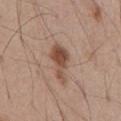This lesion was catalogued during total-body skin photography and was not selected for biopsy. A 15 mm close-up extracted from a 3D total-body photography capture. This is a white-light tile. The patient is a male aged 58 to 62. On the abdomen. Automated image analysis of the tile measured a mean CIELAB color near L≈49 a*≈20 b*≈28 and a normalized lesion–skin contrast near 9. And it measured a border-irregularity rating of about 5/10, a within-lesion color-variation index near 3/10, and a peripheral color-asymmetry measure near 1. It also reported a nevus-likeness score of about 95/100.